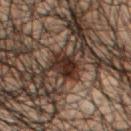Notes:
– workup · catalogued during a skin exam; not biopsied
– illumination · cross-polarized
– anatomic site · the mid back
– TBP lesion metrics · a footprint of about 6 mm² and a symmetry-axis asymmetry near 0.2; a border-irregularity index near 2/10, a color-variation rating of about 2.5/10, and radial color variation of about 1; a nevus-likeness score of about 95/100 and a detector confidence of about 90 out of 100 that the crop contains a lesion
– acquisition · ~15 mm crop, total-body skin-cancer survey
– lesion size · ~3 mm (longest diameter)
– patient · male, aged 48–52